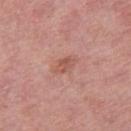| feature | finding |
|---|---|
| workup | imaged on a skin check; not biopsied |
| site | the right thigh |
| acquisition | 15 mm crop, total-body photography |
| lighting | white-light |
| size | ≈3 mm |
| patient | female, about 70 years old |